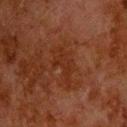Clinical impression: Recorded during total-body skin imaging; not selected for excision or biopsy. Clinical summary: A male subject, aged 78 to 82. From the head or neck. A 15 mm close-up extracted from a 3D total-body photography capture. Captured under cross-polarized illumination. Longest diameter approximately 5 mm.